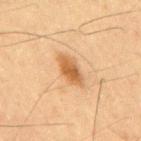Clinical impression:
Recorded during total-body skin imaging; not selected for excision or biopsy.
Background:
Captured under cross-polarized illumination. The total-body-photography lesion software estimated a border-irregularity rating of about 3/10, internal color variation of about 3.5 on a 0–10 scale, and radial color variation of about 1. A 15 mm close-up extracted from a 3D total-body photography capture. The lesion's longest dimension is about 4.5 mm. The subject is a male approximately 60 years of age.